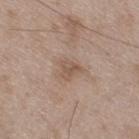workup=catalogued during a skin exam; not biopsied
diameter=≈2.5 mm
image-analysis metrics=a lesion color around L≈54 a*≈16 b*≈27 in CIELAB and a normalized border contrast of about 6; a classifier nevus-likeness of about 0/100 and a lesion-detection confidence of about 100/100
location=the right thigh
patient=male, aged 48 to 52
image source=~15 mm tile from a whole-body skin photo
lighting=white-light illumination